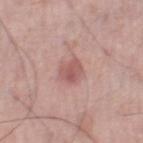biopsy_status: not biopsied; imaged during a skin examination
lesion_size:
  long_diameter_mm_approx: 2.5
lighting: white-light
image:
  source: total-body photography crop
  field_of_view_mm: 15
site: leg
patient:
  sex: male
  age_approx: 70